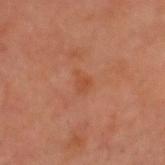Part of a total-body skin-imaging series; this lesion was reviewed on a skin check and was not flagged for biopsy. The lesion is located on the head or neck. A 15 mm close-up tile from a total-body photography series done for melanoma screening. A male subject, aged approximately 50.